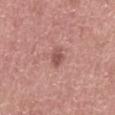workup: total-body-photography surveillance lesion; no biopsy | anatomic site: the abdomen | lesion diameter: ≈2.5 mm | image-analysis metrics: a footprint of about 3.5 mm², an outline eccentricity of about 0.75 (0 = round, 1 = elongated), and a shape-asymmetry score of about 0.25 (0 = symmetric); a nevus-likeness score of about 5/100 and a detector confidence of about 100 out of 100 that the crop contains a lesion | imaging modality: total-body-photography crop, ~15 mm field of view | lighting: white-light | subject: male, aged approximately 60.